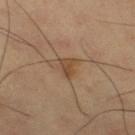No biopsy was performed on this lesion — it was imaged during a full skin examination and was not determined to be concerning. The subject is a male aged around 60. This is a cross-polarized tile. A lesion tile, about 15 mm wide, cut from a 3D total-body photograph. The lesion is on the right thigh. Longest diameter approximately 2.5 mm.